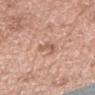{"biopsy_status": "not biopsied; imaged during a skin examination", "site": "right forearm", "image": {"source": "total-body photography crop", "field_of_view_mm": 15}, "patient": {"sex": "female", "age_approx": 60}}